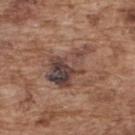No biopsy was performed on this lesion — it was imaged during a full skin examination and was not determined to be concerning. Longest diameter approximately 6 mm. This image is a 15 mm lesion crop taken from a total-body photograph. The tile uses white-light illumination. The patient is a male aged 73 to 77. On the upper back. Automated tile analysis of the lesion measured a border-irregularity index near 6/10, a within-lesion color-variation index near 10/10, and peripheral color asymmetry of about 3.5. And it measured a classifier nevus-likeness of about 0/100 and a detector confidence of about 100 out of 100 that the crop contains a lesion.